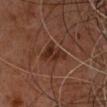Recorded during total-body skin imaging; not selected for excision or biopsy.
The tile uses cross-polarized illumination.
Longest diameter approximately 4 mm.
The total-body-photography lesion software estimated an area of roughly 6.5 mm², an outline eccentricity of about 0.8 (0 = round, 1 = elongated), and a shape-asymmetry score of about 0.45 (0 = symmetric). The analysis additionally found an average lesion color of about L≈25 a*≈19 b*≈24 (CIELAB), roughly 7 lightness units darker than nearby skin, and a lesion-to-skin contrast of about 8 (normalized; higher = more distinct).
A male subject aged 58 to 62.
A lesion tile, about 15 mm wide, cut from a 3D total-body photograph.
On the back.